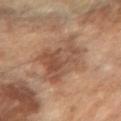| feature | finding |
|---|---|
| notes | catalogued during a skin exam; not biopsied |
| site | the right forearm |
| size | ~7.5 mm (longest diameter) |
| patient | female, aged approximately 70 |
| imaging modality | ~15 mm crop, total-body skin-cancer survey |